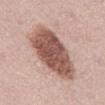{"biopsy_status": "not biopsied; imaged during a skin examination", "lighting": "white-light", "automated_metrics": {"area_mm2_approx": 31.0, "eccentricity": 0.8, "shape_asymmetry": 0.15, "cielab_L": 55, "cielab_a": 21, "cielab_b": 25, "vs_skin_darker_L": 17.0, "vs_skin_contrast_norm": 11.0, "nevus_likeness_0_100": 85}, "site": "front of the torso", "lesion_size": {"long_diameter_mm_approx": 8.5}, "image": {"source": "total-body photography crop", "field_of_view_mm": 15}, "patient": {"sex": "male", "age_approx": 55}}